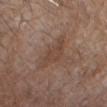| key | value |
|---|---|
| biopsy status | imaged on a skin check; not biopsied |
| acquisition | ~15 mm tile from a whole-body skin photo |
| diameter | about 4.5 mm |
| illumination | white-light |
| TBP lesion metrics | a lesion color around L≈44 a*≈17 b*≈26 in CIELAB and a normalized lesion–skin contrast near 5.5; a nevus-likeness score of about 0/100 and a lesion-detection confidence of about 100/100 |
| body site | the head or neck |
| patient | male, about 70 years old |